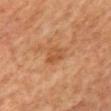| key | value |
|---|---|
| biopsy status | imaged on a skin check; not biopsied |
| image-analysis metrics | an average lesion color of about L≈42 a*≈20 b*≈32 (CIELAB), roughly 6 lightness units darker than nearby skin, and a normalized lesion–skin contrast near 5.5; a border-irregularity index near 2.5/10; an automated nevus-likeness rating near 0 out of 100 and a lesion-detection confidence of about 100/100 |
| tile lighting | cross-polarized |
| diameter | ~2.5 mm (longest diameter) |
| subject | female, aged approximately 60 |
| body site | the mid back |
| acquisition | ~15 mm tile from a whole-body skin photo |